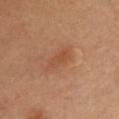Q: Was this lesion biopsied?
A: no biopsy performed (imaged during a skin exam)
Q: Where on the body is the lesion?
A: the chest
Q: What are the patient's age and sex?
A: male, roughly 55 years of age
Q: How large is the lesion?
A: ~3.5 mm (longest diameter)
Q: How was this image acquired?
A: ~15 mm crop, total-body skin-cancer survey
Q: Automated lesion metrics?
A: an average lesion color of about L≈50 a*≈25 b*≈34 (CIELAB), a lesion–skin lightness drop of about 6, and a normalized border contrast of about 5; border irregularity of about 3 on a 0–10 scale and a peripheral color-asymmetry measure near 1